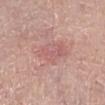| key | value |
|---|---|
| notes | total-body-photography surveillance lesion; no biopsy |
| lesion size | ≈5 mm |
| location | the right lower leg |
| illumination | white-light illumination |
| patient | male, in their 70s |
| acquisition | ~15 mm crop, total-body skin-cancer survey |